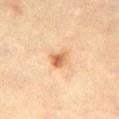Q: Was a biopsy performed?
A: no biopsy performed (imaged during a skin exam)
Q: Automated lesion metrics?
A: an area of roughly 4 mm², a shape eccentricity near 0.65, and a shape-asymmetry score of about 0.3 (0 = symmetric); a lesion color around L≈57 a*≈21 b*≈36 in CIELAB, about 11 CIELAB-L* units darker than the surrounding skin, and a normalized lesion–skin contrast near 8; border irregularity of about 2.5 on a 0–10 scale and internal color variation of about 5.5 on a 0–10 scale
Q: What are the patient's age and sex?
A: female, aged 43 to 47
Q: How was this image acquired?
A: 15 mm crop, total-body photography
Q: What lighting was used for the tile?
A: cross-polarized
Q: How large is the lesion?
A: about 2.5 mm
Q: What is the anatomic site?
A: the right thigh